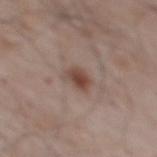<case>
  <biopsy_status>not biopsied; imaged during a skin examination</biopsy_status>
  <automated_metrics>
    <area_mm2_approx>5.5</area_mm2_approx>
    <eccentricity>0.75</eccentricity>
    <shape_asymmetry>0.25</shape_asymmetry>
    <nevus_likeness_0_100>90</nevus_likeness_0_100>
  </automated_metrics>
  <image>
    <source>total-body photography crop</source>
    <field_of_view_mm>15</field_of_view_mm>
  </image>
  <site>upper back</site>
  <patient>
    <sex>male</sex>
    <age_approx>65</age_approx>
  </patient>
</case>